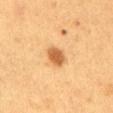follow-up — catalogued during a skin exam; not biopsied | lesion size — ~2.5 mm (longest diameter) | subject — male, aged around 55 | site — the abdomen | image-analysis metrics — a mean CIELAB color near L≈54 a*≈22 b*≈41, a lesion–skin lightness drop of about 13, and a lesion-to-skin contrast of about 9 (normalized; higher = more distinct); a border-irregularity index near 1.5/10, a within-lesion color-variation index near 2/10, and radial color variation of about 0.5 | illumination — cross-polarized illumination | imaging modality — total-body-photography crop, ~15 mm field of view.